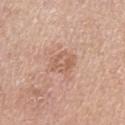Impression: Imaged during a routine full-body skin examination; the lesion was not biopsied and no histopathology is available. Image and clinical context: Captured under white-light illumination. A male subject, aged approximately 75. Approximately 3.5 mm at its widest. A region of skin cropped from a whole-body photographic capture, roughly 15 mm wide. From the left upper arm.